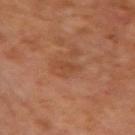Imaged during a routine full-body skin examination; the lesion was not biopsied and no histopathology is available.
Captured under cross-polarized illumination.
A male patient, about 65 years old.
A close-up tile cropped from a whole-body skin photograph, about 15 mm across.
About 2.5 mm across.
Located on the right upper arm.
An algorithmic analysis of the crop reported a border-irregularity index near 4/10, a within-lesion color-variation index near 1/10, and peripheral color asymmetry of about 0.5. It also reported a classifier nevus-likeness of about 0/100.